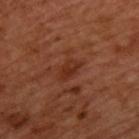Imaged during a routine full-body skin examination; the lesion was not biopsied and no histopathology is available.
On the upper back.
Captured under cross-polarized illumination.
Longest diameter approximately 3 mm.
The total-body-photography lesion software estimated a lesion area of about 4 mm², an outline eccentricity of about 0.8 (0 = round, 1 = elongated), and two-axis asymmetry of about 0.3. The software also gave roughly 7 lightness units darker than nearby skin and a lesion-to-skin contrast of about 7 (normalized; higher = more distinct). The analysis additionally found an automated nevus-likeness rating near 0 out of 100 and a detector confidence of about 100 out of 100 that the crop contains a lesion.
This image is a 15 mm lesion crop taken from a total-body photograph.
A male patient about 50 years old.